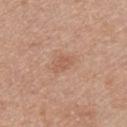Captured during whole-body skin photography for melanoma surveillance; the lesion was not biopsied.
Located on the front of the torso.
Automated tile analysis of the lesion measured a footprint of about 3 mm² and an eccentricity of roughly 0.8. It also reported border irregularity of about 3.5 on a 0–10 scale, internal color variation of about 0.5 on a 0–10 scale, and radial color variation of about 0.
A 15 mm close-up tile from a total-body photography series done for melanoma screening.
The subject is a male roughly 30 years of age.
Captured under white-light illumination.